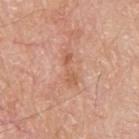This lesion was catalogued during total-body skin photography and was not selected for biopsy. A roughly 15 mm field-of-view crop from a total-body skin photograph. The subject is a male roughly 80 years of age. About 4 mm across. The lesion-visualizer software estimated a lesion area of about 4.5 mm², a shape eccentricity near 0.95, and a symmetry-axis asymmetry near 0.3. The analysis additionally found a classifier nevus-likeness of about 0/100 and a lesion-detection confidence of about 100/100. Captured under white-light illumination. On the back.